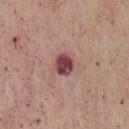Notes:
– follow-up — imaged on a skin check; not biopsied
– subject — male, in their mid- to late 50s
– acquisition — ~15 mm crop, total-body skin-cancer survey
– illumination — white-light illumination
– body site — the front of the torso